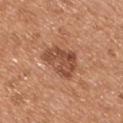The lesion was tiled from a total-body skin photograph and was not biopsied. Approximately 5 mm at its widest. A male patient, approximately 55 years of age. Captured under white-light illumination. Cropped from a whole-body photographic skin survey; the tile spans about 15 mm. Automated tile analysis of the lesion measured roughly 11 lightness units darker than nearby skin and a lesion-to-skin contrast of about 8 (normalized; higher = more distinct). It also reported a nevus-likeness score of about 15/100 and lesion-presence confidence of about 100/100. The lesion is located on the chest.